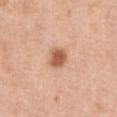Captured during whole-body skin photography for melanoma surveillance; the lesion was not biopsied. A female subject, in their 40s. Approximately 2.5 mm at its widest. Located on the chest. A 15 mm close-up tile from a total-body photography series done for melanoma screening.